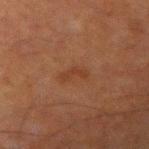Captured during whole-body skin photography for melanoma surveillance; the lesion was not biopsied. Approximately 3 mm at its widest. The patient is a male aged 58 to 62. Automated tile analysis of the lesion measured a footprint of about 3 mm² and two-axis asymmetry of about 0.5. It also reported a lesion color around L≈29 a*≈19 b*≈26 in CIELAB and about 5 CIELAB-L* units darker than the surrounding skin. And it measured a color-variation rating of about 0/10 and radial color variation of about 0. And it measured a nevus-likeness score of about 10/100. On the left arm. Cropped from a total-body skin-imaging series; the visible field is about 15 mm.